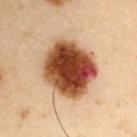Q: Is there a histopathology result?
A: imaged on a skin check; not biopsied
Q: Lesion location?
A: the left upper arm
Q: How was this image acquired?
A: ~15 mm tile from a whole-body skin photo
Q: How large is the lesion?
A: ~6.5 mm (longest diameter)
Q: Patient demographics?
A: male, aged 53 to 57